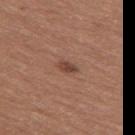Q: Was a biopsy performed?
A: total-body-photography surveillance lesion; no biopsy
Q: What lighting was used for the tile?
A: white-light illumination
Q: Patient demographics?
A: female, approximately 35 years of age
Q: What kind of image is this?
A: total-body-photography crop, ~15 mm field of view
Q: How large is the lesion?
A: ~2.5 mm (longest diameter)
Q: What is the anatomic site?
A: the left thigh